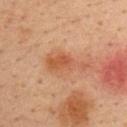Captured during whole-body skin photography for melanoma surveillance; the lesion was not biopsied.
The patient is a male in their mid- to late 30s.
The lesion is located on the upper back.
The lesion's longest dimension is about 5.5 mm.
Automated tile analysis of the lesion measured an average lesion color of about L≈51 a*≈24 b*≈34 (CIELAB), about 8 CIELAB-L* units darker than the surrounding skin, and a normalized border contrast of about 6.5. The software also gave lesion-presence confidence of about 100/100.
A 15 mm close-up tile from a total-body photography series done for melanoma screening.
This is a cross-polarized tile.